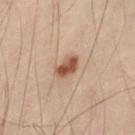Impression:
The lesion was tiled from a total-body skin photograph and was not biopsied.
Clinical summary:
A 15 mm crop from a total-body photograph taken for skin-cancer surveillance. Imaged with cross-polarized lighting. The lesion-visualizer software estimated a border-irregularity rating of about 2/10. The software also gave an automated nevus-likeness rating near 100 out of 100. Approximately 3 mm at its widest. The patient is a male aged 48 to 52. The lesion is on the abdomen.